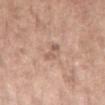workup: no biopsy performed (imaged during a skin exam)
subject: female, approximately 65 years of age
anatomic site: the left upper arm
image: ~15 mm crop, total-body skin-cancer survey
diameter: about 3 mm